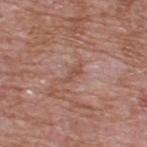Case summary:
* illumination: white-light
* patient: male, approximately 60 years of age
* site: the upper back
* size: ~2.5 mm (longest diameter)
* image source: 15 mm crop, total-body photography
* automated lesion analysis: a lesion color around L≈51 a*≈22 b*≈27 in CIELAB, roughly 7 lightness units darker than nearby skin, and a lesion-to-skin contrast of about 5.5 (normalized; higher = more distinct)